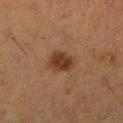| key | value |
|---|---|
| workup | total-body-photography surveillance lesion; no biopsy |
| lesion diameter | ≈3.5 mm |
| location | the leg |
| subject | female, approximately 50 years of age |
| acquisition | total-body-photography crop, ~15 mm field of view |